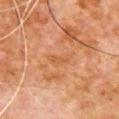<lesion>
<biopsy_status>not biopsied; imaged during a skin examination</biopsy_status>
<patient>
  <sex>male</sex>
  <age_approx>80</age_approx>
</patient>
<lesion_size>
  <long_diameter_mm_approx>3.5</long_diameter_mm_approx>
</lesion_size>
<automated_metrics>
  <cielab_L>44</cielab_L>
  <cielab_a>21</cielab_a>
  <cielab_b>33</cielab_b>
  <vs_skin_darker_L>4.0</vs_skin_darker_L>
</automated_metrics>
<image>
  <source>total-body photography crop</source>
  <field_of_view_mm>15</field_of_view_mm>
</image>
<site>chest</site>
<lighting>cross-polarized</lighting>
</lesion>